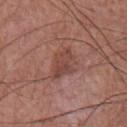Assessment: The lesion was photographed on a routine skin check and not biopsied; there is no pathology result. Image and clinical context: Longest diameter approximately 4 mm. The patient is a male aged approximately 75. Captured under white-light illumination. From the chest. A lesion tile, about 15 mm wide, cut from a 3D total-body photograph.